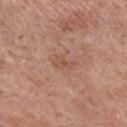Notes:
• notes — catalogued during a skin exam; not biopsied
• image — total-body-photography crop, ~15 mm field of view
• automated metrics — a lesion area of about 3.5 mm², an outline eccentricity of about 0.85 (0 = round, 1 = elongated), and a symmetry-axis asymmetry near 0.35; an average lesion color of about L≈53 a*≈22 b*≈28 (CIELAB), roughly 6 lightness units darker than nearby skin, and a normalized lesion–skin contrast near 4.5; a border-irregularity index near 3.5/10, a within-lesion color-variation index near 1/10, and radial color variation of about 0.5
• patient — male, aged around 75
• site — the right lower leg